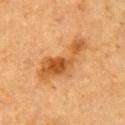Imaged during a routine full-body skin examination; the lesion was not biopsied and no histopathology is available. The recorded lesion diameter is about 7 mm. A close-up tile cropped from a whole-body skin photograph, about 15 mm across. A male subject, roughly 85 years of age or older. On the right upper arm.